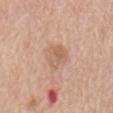Cropped from a whole-body photographic skin survey; the tile spans about 15 mm.
Located on the mid back.
A male subject in their mid-60s.